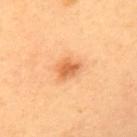This lesion was catalogued during total-body skin photography and was not selected for biopsy.